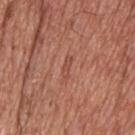The lesion was photographed on a routine skin check and not biopsied; there is no pathology result. Imaged with white-light lighting. Cropped from a whole-body photographic skin survey; the tile spans about 15 mm. From the head or neck. The subject is a male aged around 65. Longest diameter approximately 2.5 mm.